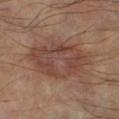• follow-up · imaged on a skin check; not biopsied
• subject · male, aged around 60
• tile lighting · cross-polarized
• imaging modality · ~15 mm crop, total-body skin-cancer survey
• diameter · about 7.5 mm
• anatomic site · the leg